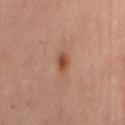Impression: No biopsy was performed on this lesion — it was imaged during a full skin examination and was not determined to be concerning. Image and clinical context: A 15 mm close-up extracted from a 3D total-body photography capture. The patient is a female about 55 years old. Automated tile analysis of the lesion measured border irregularity of about 2 on a 0–10 scale, internal color variation of about 1.5 on a 0–10 scale, and radial color variation of about 0.5. The lesion is located on the mid back. This is a cross-polarized tile. The lesion's longest dimension is about 2.5 mm.